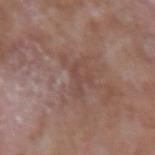The lesion was tiled from a total-body skin photograph and was not biopsied.
From the left upper arm.
A male subject, roughly 65 years of age.
A lesion tile, about 15 mm wide, cut from a 3D total-body photograph.
Measured at roughly 2.5 mm in maximum diameter.
The total-body-photography lesion software estimated an average lesion color of about L≈45 a*≈20 b*≈23 (CIELAB) and a lesion–skin lightness drop of about 6. The analysis additionally found a classifier nevus-likeness of about 0/100 and a lesion-detection confidence of about 95/100.
This is a white-light tile.